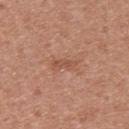Notes:
* workup: no biopsy performed (imaged during a skin exam)
* image source: ~15 mm crop, total-body skin-cancer survey
* tile lighting: white-light illumination
* site: the upper back
* subject: male, in their 30s
* lesion size: ≈3 mm
* image-analysis metrics: an area of roughly 3 mm² and two-axis asymmetry of about 0.35